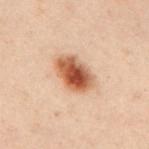biopsy status: total-body-photography surveillance lesion; no biopsy
image source: total-body-photography crop, ~15 mm field of view
diameter: ~5 mm (longest diameter)
tile lighting: cross-polarized
body site: the mid back
patient: male, roughly 50 years of age